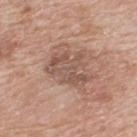workup: total-body-photography surveillance lesion; no biopsy | patient: male, aged around 65 | location: the upper back | image: total-body-photography crop, ~15 mm field of view.